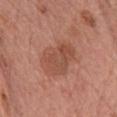Clinical impression:
Part of a total-body skin-imaging series; this lesion was reviewed on a skin check and was not flagged for biopsy.
Image and clinical context:
Cropped from a whole-body photographic skin survey; the tile spans about 15 mm. From the chest. About 5.5 mm across. A female patient approximately 60 years of age. This is a white-light tile.